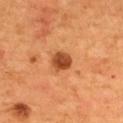Impression: No biopsy was performed on this lesion — it was imaged during a full skin examination and was not determined to be concerning. Background: The lesion's longest dimension is about 2.5 mm. Imaged with cross-polarized lighting. The lesion is located on the mid back. A male subject, aged around 55. A region of skin cropped from a whole-body photographic capture, roughly 15 mm wide.